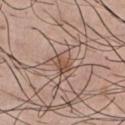The lesion was tiled from a total-body skin photograph and was not biopsied. This is a white-light tile. The lesion is on the chest. Cropped from a whole-body photographic skin survey; the tile spans about 15 mm. About 3 mm across. A male patient about 30 years old.